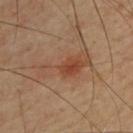Acquisition and patient details:
A close-up tile cropped from a whole-body skin photograph, about 15 mm across. An algorithmic analysis of the crop reported an average lesion color of about L≈45 a*≈21 b*≈31 (CIELAB) and a lesion–skin lightness drop of about 8. And it measured a within-lesion color-variation index near 4.5/10 and a peripheral color-asymmetry measure near 1. And it measured a classifier nevus-likeness of about 70/100. Longest diameter approximately 6.5 mm. Captured under cross-polarized illumination. From the upper back. The subject is a male approximately 65 years of age.